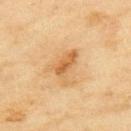Impression:
The lesion was photographed on a routine skin check and not biopsied; there is no pathology result.
Acquisition and patient details:
Imaged with cross-polarized lighting. Located on the upper back. A female subject, approximately 60 years of age. The lesion's longest dimension is about 4.5 mm. A 15 mm crop from a total-body photograph taken for skin-cancer surveillance.